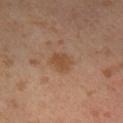biopsy_status: not biopsied; imaged during a skin examination
site: left leg
image:
  source: total-body photography crop
  field_of_view_mm: 15
patient:
  sex: male
  age_approx: 30